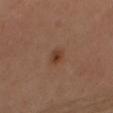Imaged during a routine full-body skin examination; the lesion was not biopsied and no histopathology is available.
Captured under cross-polarized illumination.
A female subject, aged 53–57.
The lesion is located on the right thigh.
This image is a 15 mm lesion crop taken from a total-body photograph.
The recorded lesion diameter is about 2.5 mm.